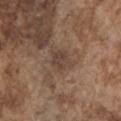Image and clinical context: A 15 mm close-up tile from a total-body photography series done for melanoma screening. The patient is a male about 75 years old. On the left upper arm.